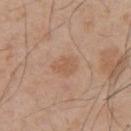biopsy_status: not biopsied; imaged during a skin examination
image:
  source: total-body photography crop
  field_of_view_mm: 15
lesion_size:
  long_diameter_mm_approx: 3.0
site: back
lighting: white-light
patient:
  sex: male
  age_approx: 55